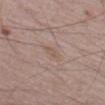The lesion was photographed on a routine skin check and not biopsied; there is no pathology result.
On the left thigh.
Automated tile analysis of the lesion measured an outline eccentricity of about 0.85 (0 = round, 1 = elongated) and a shape-asymmetry score of about 0.2 (0 = symmetric). The software also gave a border-irregularity index near 2/10, internal color variation of about 2 on a 0–10 scale, and radial color variation of about 0.5. And it measured a nevus-likeness score of about 0/100.
A 15 mm close-up tile from a total-body photography series done for melanoma screening.
The patient is a male aged approximately 75.